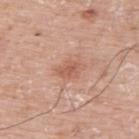No biopsy was performed on this lesion — it was imaged during a full skin examination and was not determined to be concerning.
A male patient approximately 75 years of age.
Measured at roughly 2.5 mm in maximum diameter.
Imaged with white-light lighting.
A roughly 15 mm field-of-view crop from a total-body skin photograph.
On the upper back.
The total-body-photography lesion software estimated an area of roughly 4 mm², a shape eccentricity near 0.75, and a symmetry-axis asymmetry near 0.3. And it measured about 9 CIELAB-L* units darker than the surrounding skin. And it measured border irregularity of about 2.5 on a 0–10 scale, a color-variation rating of about 2/10, and a peripheral color-asymmetry measure near 0.5. And it measured a nevus-likeness score of about 0/100 and a lesion-detection confidence of about 100/100.